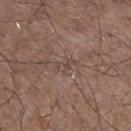Q: Was a biopsy performed?
A: imaged on a skin check; not biopsied
Q: What is the lesion's diameter?
A: about 2.5 mm
Q: Automated lesion metrics?
A: about 6 CIELAB-L* units darker than the surrounding skin and a normalized border contrast of about 4.5; border irregularity of about 4 on a 0–10 scale, a within-lesion color-variation index near 0/10, and peripheral color asymmetry of about 0
Q: Lesion location?
A: the left lower leg
Q: How was this image acquired?
A: total-body-photography crop, ~15 mm field of view
Q: How was the tile lit?
A: white-light illumination
Q: Patient demographics?
A: male, approximately 60 years of age